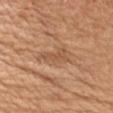follow-up: total-body-photography surveillance lesion; no biopsy | patient: female, in their mid- to late 40s | size: about 4 mm | imaging modality: total-body-photography crop, ~15 mm field of view | lighting: white-light | anatomic site: the arm | automated metrics: a footprint of about 5.5 mm², a shape eccentricity near 0.9, and a shape-asymmetry score of about 0.4 (0 = symmetric); border irregularity of about 5 on a 0–10 scale, a within-lesion color-variation index near 1.5/10, and peripheral color asymmetry of about 0.5.